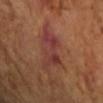Clinical impression:
The lesion was photographed on a routine skin check and not biopsied; there is no pathology result.
Acquisition and patient details:
Captured under cross-polarized illumination. A 15 mm close-up tile from a total-body photography series done for melanoma screening. The patient is a female about 70 years old. An algorithmic analysis of the crop reported a border-irregularity rating of about 8/10 and internal color variation of about 3.5 on a 0–10 scale. The software also gave lesion-presence confidence of about 100/100. The lesion is located on the right forearm.